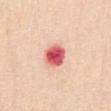follow-up: total-body-photography surveillance lesion; no biopsy
illumination: white-light illumination
lesion diameter: ≈3 mm
imaging modality: 15 mm crop, total-body photography
subject: female, about 55 years old
automated metrics: an area of roughly 7 mm², an eccentricity of roughly 0.25, and a shape-asymmetry score of about 0.15 (0 = symmetric); an average lesion color of about L≈61 a*≈41 b*≈28 (CIELAB) and roughly 20 lightness units darker than nearby skin; a border-irregularity index near 1/10 and a color-variation rating of about 5/10; a nevus-likeness score of about 0/100 and a detector confidence of about 100 out of 100 that the crop contains a lesion
site: the front of the torso